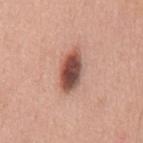biopsy status — no biopsy performed (imaged during a skin exam)
subject — male, aged 58–62
imaging modality — 15 mm crop, total-body photography
anatomic site — the mid back